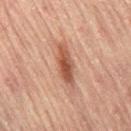follow-up = imaged on a skin check; not biopsied
body site = the left leg
patient = female, aged 78 to 82
size = ≈5 mm
automated metrics = a normalized lesion–skin contrast near 9; border irregularity of about 3 on a 0–10 scale and a color-variation rating of about 3.5/10; an automated nevus-likeness rating near 95 out of 100 and a lesion-detection confidence of about 100/100
imaging modality = 15 mm crop, total-body photography
tile lighting = cross-polarized